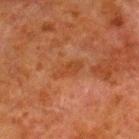Case summary:
- follow-up: imaged on a skin check; not biopsied
- imaging modality: 15 mm crop, total-body photography
- size: ≈3.5 mm
- anatomic site: the right lower leg
- subject: male, in their 80s
- illumination: cross-polarized